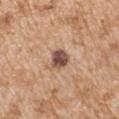workup: total-body-photography surveillance lesion; no biopsy
patient: male, in their mid- to late 60s
tile lighting: white-light illumination
diameter: ≈3 mm
acquisition: ~15 mm crop, total-body skin-cancer survey
anatomic site: the left upper arm